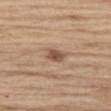Located on the left thigh.
The patient is a male about 70 years old.
Cropped from a total-body skin-imaging series; the visible field is about 15 mm.
This is a white-light tile.
The recorded lesion diameter is about 3 mm.